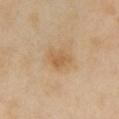<case>
  <biopsy_status>not biopsied; imaged during a skin examination</biopsy_status>
  <lesion_size>
    <long_diameter_mm_approx>2.5</long_diameter_mm_approx>
  </lesion_size>
  <image>
    <source>total-body photography crop</source>
    <field_of_view_mm>15</field_of_view_mm>
  </image>
  <automated_metrics>
    <area_mm2_approx>4.5</area_mm2_approx>
    <cielab_L>59</cielab_L>
    <cielab_a>18</cielab_a>
    <cielab_b>38</cielab_b>
    <vs_skin_darker_L>8.0</vs_skin_darker_L>
  </automated_metrics>
  <patient>
    <sex>female</sex>
    <age_approx>55</age_approx>
  </patient>
  <site>front of the torso</site>
  <lighting>cross-polarized</lighting>
</case>